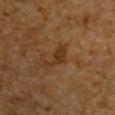follow-up=imaged on a skin check; not biopsied